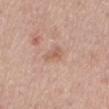  biopsy_status: not biopsied; imaged during a skin examination
  image:
    source: total-body photography crop
    field_of_view_mm: 15
  lighting: white-light
  patient:
    sex: female
    age_approx: 60
  automated_metrics:
    area_mm2_approx: 4.0
    eccentricity: 0.85
    shape_asymmetry: 0.35
    cielab_L: 60
    cielab_a: 19
    cielab_b: 29
  lesion_size:
    long_diameter_mm_approx: 3.0
  site: back